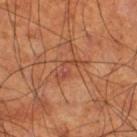workup: catalogued during a skin exam; not biopsied
size: ≈4 mm
imaging modality: total-body-photography crop, ~15 mm field of view
location: the right thigh
image-analysis metrics: a shape eccentricity near 0.9
patient: male, about 70 years old